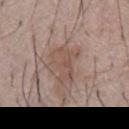The lesion was tiled from a total-body skin photograph and was not biopsied.
A roughly 15 mm field-of-view crop from a total-body skin photograph.
The subject is a male aged 73–77.
The lesion is located on the chest.